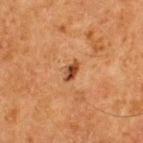| key | value |
|---|---|
| follow-up | catalogued during a skin exam; not biopsied |
| acquisition | ~15 mm crop, total-body skin-cancer survey |
| illumination | cross-polarized illumination |
| body site | the upper back |
| lesion size | ~2.5 mm (longest diameter) |
| patient | male, aged 63 to 67 |